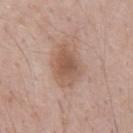The lesion was tiled from a total-body skin photograph and was not biopsied. From the chest. Measured at roughly 5.5 mm in maximum diameter. The subject is a male aged 68–72. Automated image analysis of the tile measured an area of roughly 14 mm², a shape eccentricity near 0.75, and two-axis asymmetry of about 0.2. The analysis additionally found about 10 CIELAB-L* units darker than the surrounding skin. A region of skin cropped from a whole-body photographic capture, roughly 15 mm wide.